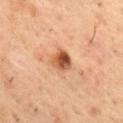Findings:
– biopsy status · no biopsy performed (imaged during a skin exam)
– diameter · ≈2.5 mm
– subject · male, roughly 55 years of age
– automated lesion analysis · a footprint of about 5.5 mm², an eccentricity of roughly 0.45, and a symmetry-axis asymmetry near 0.2; a border-irregularity index near 2/10, a color-variation rating of about 8.5/10, and radial color variation of about 3
– imaging modality · 15 mm crop, total-body photography
– tile lighting · cross-polarized
– location · the mid back